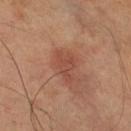follow-up — total-body-photography surveillance lesion; no biopsy
subject — male, aged approximately 65
illumination — cross-polarized
imaging modality — total-body-photography crop, ~15 mm field of view
body site — the right thigh